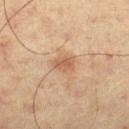Captured during whole-body skin photography for melanoma surveillance; the lesion was not biopsied.
The lesion's longest dimension is about 2.5 mm.
An algorithmic analysis of the crop reported a footprint of about 4.5 mm². And it measured a color-variation rating of about 2.5/10 and peripheral color asymmetry of about 1. The software also gave an automated nevus-likeness rating near 65 out of 100.
Imaged with cross-polarized lighting.
On the left thigh.
A roughly 15 mm field-of-view crop from a total-body skin photograph.
A male patient approximately 65 years of age.